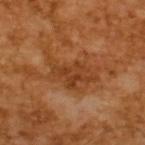Notes:
* patient · male, aged around 65
* illumination · cross-polarized illumination
* lesion size · ≈7 mm
* image-analysis metrics · a lesion area of about 16 mm², an outline eccentricity of about 0.8 (0 = round, 1 = elongated), and two-axis asymmetry of about 0.45; a mean CIELAB color near L≈40 a*≈24 b*≈37, a lesion–skin lightness drop of about 8, and a normalized border contrast of about 6.5
* image source · total-body-photography crop, ~15 mm field of view